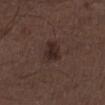Q: Is there a histopathology result?
A: total-body-photography surveillance lesion; no biopsy
Q: What is the anatomic site?
A: the left thigh
Q: How was the tile lit?
A: white-light
Q: What is the imaging modality?
A: total-body-photography crop, ~15 mm field of view
Q: What are the patient's age and sex?
A: male, in their 50s